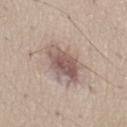Notes:
– biopsy status — catalogued during a skin exam; not biopsied
– acquisition — total-body-photography crop, ~15 mm field of view
– automated lesion analysis — a lesion area of about 13 mm², a shape eccentricity near 0.75, and two-axis asymmetry of about 0.2; a mean CIELAB color near L≈55 a*≈16 b*≈21 and about 12 CIELAB-L* units darker than the surrounding skin
– lighting — white-light
– patient — female, aged 58–62
– lesion diameter — about 5.5 mm
– anatomic site — the left thigh